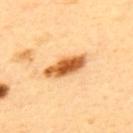No biopsy was performed on this lesion — it was imaged during a full skin examination and was not determined to be concerning.
From the upper back.
A male subject, aged 38 to 42.
A 15 mm close-up extracted from a 3D total-body photography capture.
About 5.5 mm across.